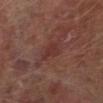Q: Was this lesion biopsied?
A: no biopsy performed (imaged during a skin exam)
Q: What is the imaging modality?
A: total-body-photography crop, ~15 mm field of view
Q: Who is the patient?
A: male, in their mid-60s
Q: What is the lesion's diameter?
A: ~3 mm (longest diameter)
Q: Lesion location?
A: the left lower leg